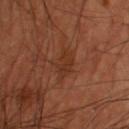Assessment: This lesion was catalogued during total-body skin photography and was not selected for biopsy. Background: The lesion is located on the back. The patient is a male about 60 years old. A 15 mm crop from a total-body photograph taken for skin-cancer surveillance.